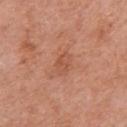Assessment: No biopsy was performed on this lesion — it was imaged during a full skin examination and was not determined to be concerning. Acquisition and patient details: The subject is a female roughly 60 years of age. From the right upper arm. A 15 mm close-up extracted from a 3D total-body photography capture. Imaged with white-light lighting.